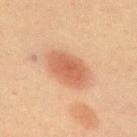Case summary:
- notes — catalogued during a skin exam; not biopsied
- acquisition — total-body-photography crop, ~15 mm field of view
- subject — male, about 60 years old
- location — the upper back